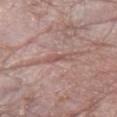{
  "biopsy_status": "not biopsied; imaged during a skin examination",
  "image": {
    "source": "total-body photography crop",
    "field_of_view_mm": 15
  },
  "patient": {
    "sex": "female",
    "age_approx": 65
  },
  "lighting": "white-light",
  "site": "left forearm"
}